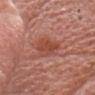Q: Was a biopsy performed?
A: total-body-photography surveillance lesion; no biopsy
Q: How was this image acquired?
A: ~15 mm crop, total-body skin-cancer survey
Q: Where on the body is the lesion?
A: the head or neck
Q: Who is the patient?
A: male, about 60 years old
Q: What did automated image analysis measure?
A: an average lesion color of about L≈48 a*≈28 b*≈30 (CIELAB) and about 9 CIELAB-L* units darker than the surrounding skin; a classifier nevus-likeness of about 80/100 and a lesion-detection confidence of about 100/100
Q: How was the tile lit?
A: white-light illumination